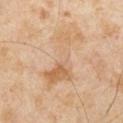Q: Is there a histopathology result?
A: no biopsy performed (imaged during a skin exam)
Q: Automated lesion metrics?
A: a color-variation rating of about 6/10 and radial color variation of about 1.5
Q: What is the imaging modality?
A: 15 mm crop, total-body photography
Q: What lighting was used for the tile?
A: cross-polarized
Q: Who is the patient?
A: male, approximately 65 years of age
Q: Lesion size?
A: ≈8 mm